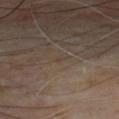The lesion was photographed on a routine skin check and not biopsied; there is no pathology result.
The patient is a male aged 63–67.
From the chest.
Cropped from a whole-body photographic skin survey; the tile spans about 15 mm.